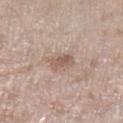Clinical impression: This lesion was catalogued during total-body skin photography and was not selected for biopsy. Clinical summary: Cropped from a total-body skin-imaging series; the visible field is about 15 mm. The patient is a female aged 73 to 77. From the leg.